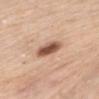notes=no biopsy performed (imaged during a skin exam); subject=female, about 65 years old; image-analysis metrics=an area of roughly 7 mm², a shape eccentricity near 0.85, and a symmetry-axis asymmetry near 0.15; imaging modality=15 mm crop, total-body photography; tile lighting=white-light illumination; site=the chest; lesion diameter=~4 mm (longest diameter).